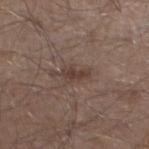Q: What lighting was used for the tile?
A: white-light illumination
Q: How large is the lesion?
A: about 3 mm
Q: Patient demographics?
A: male, aged 53–57
Q: How was this image acquired?
A: ~15 mm crop, total-body skin-cancer survey
Q: Where on the body is the lesion?
A: the left lower leg
Q: What did automated image analysis measure?
A: a footprint of about 3.5 mm²; a nevus-likeness score of about 5/100 and lesion-presence confidence of about 100/100